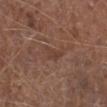illumination: white-light illumination
subject: male, aged 73 to 77
lesion size: about 3 mm
TBP lesion metrics: an outline eccentricity of about 0.9 (0 = round, 1 = elongated) and a shape-asymmetry score of about 0.45 (0 = symmetric); a classifier nevus-likeness of about 0/100
image: ~15 mm crop, total-body skin-cancer survey
anatomic site: the leg